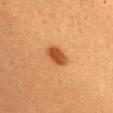Acquisition and patient details: The subject is a female aged approximately 30. This image is a 15 mm lesion crop taken from a total-body photograph. On the head or neck. Imaged with cross-polarized lighting. Measured at roughly 3.5 mm in maximum diameter.